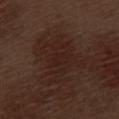Cropped from a whole-body photographic skin survey; the tile spans about 15 mm. The tile uses white-light illumination. On the left thigh. Approximately 6 mm at its widest. A male subject, aged approximately 70.